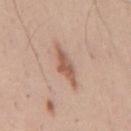biopsy status: catalogued during a skin exam; not biopsied | imaging modality: ~15 mm tile from a whole-body skin photo | subject: male, aged 38–42 | body site: the mid back.